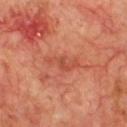No biopsy was performed on this lesion — it was imaged during a full skin examination and was not determined to be concerning.
A 15 mm close-up tile from a total-body photography series done for melanoma screening.
This is a cross-polarized tile.
A male patient, approximately 70 years of age.
On the chest.
Automated tile analysis of the lesion measured a footprint of about 5.5 mm², an eccentricity of roughly 0.9, and two-axis asymmetry of about 0.4.